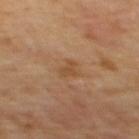No biopsy was performed on this lesion — it was imaged during a full skin examination and was not determined to be concerning. Measured at roughly 3 mm in maximum diameter. On the mid back. The tile uses cross-polarized illumination. A male patient aged 58 to 62. A 15 mm close-up tile from a total-body photography series done for melanoma screening.